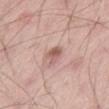– follow-up — catalogued during a skin exam; not biopsied
– imaging modality — ~15 mm tile from a whole-body skin photo
– illumination — white-light illumination
– location — the left thigh
– subject — male, about 55 years old